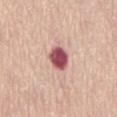Imaged during a routine full-body skin examination; the lesion was not biopsied and no histopathology is available. The lesion is on the front of the torso. The tile uses white-light illumination. A lesion tile, about 15 mm wide, cut from a 3D total-body photograph. A female patient, aged around 70. Automated tile analysis of the lesion measured a mean CIELAB color near L≈53 a*≈30 b*≈20, about 20 CIELAB-L* units darker than the surrounding skin, and a lesion-to-skin contrast of about 13 (normalized; higher = more distinct). The software also gave a border-irregularity index near 1.5/10, internal color variation of about 4 on a 0–10 scale, and radial color variation of about 1. The analysis additionally found a classifier nevus-likeness of about 0/100.